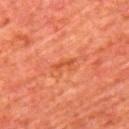<tbp_lesion>
<biopsy_status>not biopsied; imaged during a skin examination</biopsy_status>
<image>
  <source>total-body photography crop</source>
  <field_of_view_mm>15</field_of_view_mm>
</image>
<lighting>cross-polarized</lighting>
<lesion_size>
  <long_diameter_mm_approx>2.5</long_diameter_mm_approx>
</lesion_size>
<patient>
  <sex>male</sex>
  <age_approx>65</age_approx>
</patient>
<site>upper back</site>
</tbp_lesion>